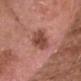Recorded during total-body skin imaging; not selected for excision or biopsy. Imaged with white-light lighting. About 6 mm across. A close-up tile cropped from a whole-body skin photograph, about 15 mm across. The subject is a male aged 73–77. Located on the head or neck.